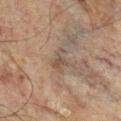Assessment:
The lesion was tiled from a total-body skin photograph and was not biopsied.
Clinical summary:
About 3 mm across. This is a cross-polarized tile. The patient is a male in their 60s. On the left leg. Cropped from a whole-body photographic skin survey; the tile spans about 15 mm.